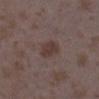  biopsy_status: not biopsied; imaged during a skin examination
  image:
    source: total-body photography crop
    field_of_view_mm: 15
  lighting: white-light
  lesion_size:
    long_diameter_mm_approx: 3.0
  site: right lower leg
  patient:
    sex: female
    age_approx: 35